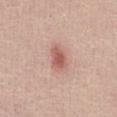Assessment:
Captured during whole-body skin photography for melanoma surveillance; the lesion was not biopsied.
Clinical summary:
The lesion-visualizer software estimated a shape eccentricity near 0.75 and two-axis asymmetry of about 0.15. And it measured a border-irregularity index near 1.5/10 and radial color variation of about 0.5. The software also gave a classifier nevus-likeness of about 80/100 and a lesion-detection confidence of about 100/100. The patient is a male about 40 years old. A 15 mm close-up extracted from a 3D total-body photography capture. Approximately 3 mm at its widest. From the front of the torso.